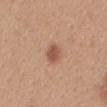Acquisition and patient details:
Captured under white-light illumination. The total-body-photography lesion software estimated an area of roughly 4.5 mm² and a shape-asymmetry score of about 0.25 (0 = symmetric). The analysis additionally found an average lesion color of about L≈53 a*≈22 b*≈30 (CIELAB) and a normalized lesion–skin contrast near 7.5. The software also gave a border-irregularity rating of about 2.5/10, internal color variation of about 1.5 on a 0–10 scale, and a peripheral color-asymmetry measure near 0.5. A female subject, in their 30s. Located on the mid back. A roughly 15 mm field-of-view crop from a total-body skin photograph.